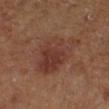Part of a total-body skin-imaging series; this lesion was reviewed on a skin check and was not flagged for biopsy.
A 15 mm crop from a total-body photograph taken for skin-cancer surveillance.
Approximately 6.5 mm at its widest.
Automated image analysis of the tile measured an average lesion color of about L≈38 a*≈22 b*≈26 (CIELAB), a lesion–skin lightness drop of about 7, and a normalized lesion–skin contrast near 6.5. The software also gave a nevus-likeness score of about 30/100 and a lesion-detection confidence of about 100/100.
Captured under cross-polarized illumination.
The patient is female.
From the right lower leg.